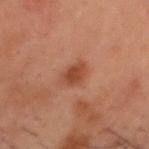Part of a total-body skin-imaging series; this lesion was reviewed on a skin check and was not flagged for biopsy. A region of skin cropped from a whole-body photographic capture, roughly 15 mm wide. The lesion is located on the head or neck. The patient is a male aged 48–52.